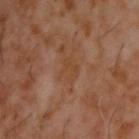| field | value |
|---|---|
| workup | total-body-photography surveillance lesion; no biopsy |
| site | the upper back |
| imaging modality | total-body-photography crop, ~15 mm field of view |
| patient | male, approximately 60 years of age |